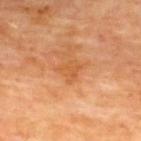Acquisition and patient details: An algorithmic analysis of the crop reported a lesion area of about 4 mm², an outline eccentricity of about 0.55 (0 = round, 1 = elongated), and a symmetry-axis asymmetry near 0.45. And it measured peripheral color asymmetry of about 0.5. Imaged with cross-polarized lighting. Located on the back. The subject is a male roughly 70 years of age. A 15 mm close-up extracted from a 3D total-body photography capture. Longest diameter approximately 3 mm.